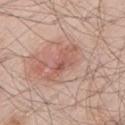| feature | finding |
|---|---|
| notes | catalogued during a skin exam; not biopsied |
| lesion diameter | ≈5.5 mm |
| patient | male, aged around 60 |
| body site | the leg |
| image | ~15 mm crop, total-body skin-cancer survey |
| tile lighting | white-light |